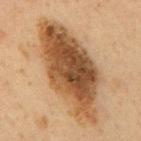The lesion was photographed on a routine skin check and not biopsied; there is no pathology result.
From the mid back.
A male patient, in their 60s.
The tile uses cross-polarized illumination.
Cropped from a whole-body photographic skin survey; the tile spans about 15 mm.